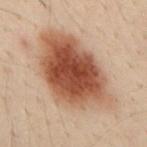Part of a total-body skin-imaging series; this lesion was reviewed on a skin check and was not flagged for biopsy. Automated image analysis of the tile measured a lesion–skin lightness drop of about 14. It also reported border irregularity of about 2.5 on a 0–10 scale, a within-lesion color-variation index near 7/10, and peripheral color asymmetry of about 2. And it measured a lesion-detection confidence of about 100/100. Cropped from a whole-body photographic skin survey; the tile spans about 15 mm. The lesion is on the mid back. A male subject, aged 28 to 32. This is a cross-polarized tile.